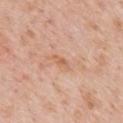Imaged during a routine full-body skin examination; the lesion was not biopsied and no histopathology is available.
A male subject, aged 58–62.
A 15 mm crop from a total-body photograph taken for skin-cancer surveillance.
Captured under white-light illumination.
Longest diameter approximately 2.5 mm.
From the mid back.